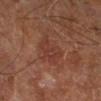The lesion was photographed on a routine skin check and not biopsied; there is no pathology result. This is a cross-polarized tile. The patient is roughly 65 years of age. The lesion is located on the right lower leg. This image is a 15 mm lesion crop taken from a total-body photograph.